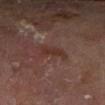* notes — no biopsy performed (imaged during a skin exam)
* automated metrics — an area of roughly 5 mm² and a shape-asymmetry score of about 0.4 (0 = symmetric)
* lesion size — ~3 mm (longest diameter)
* tile lighting — cross-polarized
* image — ~15 mm tile from a whole-body skin photo
* patient — male, approximately 70 years of age
* anatomic site — the right lower leg